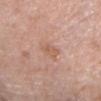Q: Was a biopsy performed?
A: imaged on a skin check; not biopsied
Q: Lesion size?
A: ~2.5 mm (longest diameter)
Q: Where on the body is the lesion?
A: the head or neck
Q: Patient demographics?
A: female, about 60 years old
Q: How was the tile lit?
A: white-light illumination
Q: What is the imaging modality?
A: ~15 mm crop, total-body skin-cancer survey